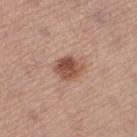Impression: This lesion was catalogued during total-body skin photography and was not selected for biopsy. Image and clinical context: Located on the left thigh. A lesion tile, about 15 mm wide, cut from a 3D total-body photograph. The recorded lesion diameter is about 3.5 mm. The patient is a female in their mid-60s. This is a white-light tile.